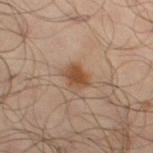About 2.5 mm across. The total-body-photography lesion software estimated an area of roughly 4 mm², a shape eccentricity near 0.65, and a symmetry-axis asymmetry near 0.25. And it measured an automated nevus-likeness rating near 95 out of 100 and a lesion-detection confidence of about 100/100. This image is a 15 mm lesion crop taken from a total-body photograph. This is a cross-polarized tile. The lesion is on the left thigh. The subject is a male about 65 years old.